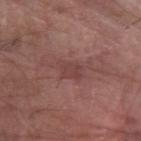Impression:
This lesion was catalogued during total-body skin photography and was not selected for biopsy.
Image and clinical context:
A roughly 15 mm field-of-view crop from a total-body skin photograph. This is a white-light tile. Longest diameter approximately 3 mm. The lesion is located on the left forearm. A male subject aged 68 to 72.